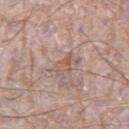Clinical impression: No biopsy was performed on this lesion — it was imaged during a full skin examination and was not determined to be concerning. Acquisition and patient details: On the right thigh. A male subject, approximately 75 years of age. Automated tile analysis of the lesion measured a mean CIELAB color near L≈56 a*≈18 b*≈25, about 7 CIELAB-L* units darker than the surrounding skin, and a normalized lesion–skin contrast near 5.5. The analysis additionally found a border-irregularity rating of about 3.5/10. This image is a 15 mm lesion crop taken from a total-body photograph. The lesion's longest dimension is about 3 mm.